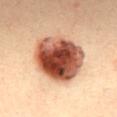Recorded during total-body skin imaging; not selected for excision or biopsy. The subject is a female about 35 years old. The lesion-visualizer software estimated a lesion area of about 31 mm², an outline eccentricity of about 0.45 (0 = round, 1 = elongated), and a symmetry-axis asymmetry near 0.1. And it measured a border-irregularity index near 1/10 and a within-lesion color-variation index near 10/10. A roughly 15 mm field-of-view crop from a total-body skin photograph. Longest diameter approximately 6.5 mm. The tile uses cross-polarized illumination. On the mid back.